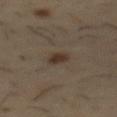Clinical impression: Captured during whole-body skin photography for melanoma surveillance; the lesion was not biopsied. Background: Cropped from a whole-body photographic skin survey; the tile spans about 15 mm. The subject is a male roughly 55 years of age. Imaged with cross-polarized lighting. The lesion-visualizer software estimated an average lesion color of about L≈32 a*≈11 b*≈22 (CIELAB), roughly 9 lightness units darker than nearby skin, and a normalized lesion–skin contrast near 8.5. It also reported a border-irregularity rating of about 2/10, a color-variation rating of about 2/10, and a peripheral color-asymmetry measure near 0.5. The lesion is located on the mid back. About 3 mm across.